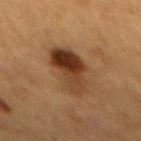The lesion was tiled from a total-body skin photograph and was not biopsied. A female patient aged around 55. A close-up tile cropped from a whole-body skin photograph, about 15 mm across. From the mid back. The lesion's longest dimension is about 6 mm. This is a cross-polarized tile.